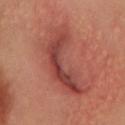Q: Is there a histopathology result?
A: no biopsy performed (imaged during a skin exam)
Q: Who is the patient?
A: female, aged approximately 35
Q: How was this image acquired?
A: total-body-photography crop, ~15 mm field of view
Q: Illumination type?
A: cross-polarized
Q: What did automated image analysis measure?
A: a lesion area of about 26 mm², a shape eccentricity near 0.9, and two-axis asymmetry of about 0.55; a lesion color around L≈44 a*≈29 b*≈26 in CIELAB, roughly 10 lightness units darker than nearby skin, and a normalized lesion–skin contrast near 7.5; an automated nevus-likeness rating near 0 out of 100 and a lesion-detection confidence of about 70/100
Q: Where on the body is the lesion?
A: the chest
Q: How large is the lesion?
A: ≈9 mm